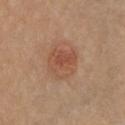follow-up — catalogued during a skin exam; not biopsied | anatomic site — the chest | lighting — white-light illumination | image source — total-body-photography crop, ~15 mm field of view | subject — female, aged 58 to 62 | image-analysis metrics — a footprint of about 11 mm², a shape eccentricity near 0.35, and a symmetry-axis asymmetry near 0.2; a mean CIELAB color near L≈51 a*≈23 b*≈32, about 7 CIELAB-L* units darker than the surrounding skin, and a normalized lesion–skin contrast near 5.5; a classifier nevus-likeness of about 80/100 and lesion-presence confidence of about 100/100 | lesion size — ~4 mm (longest diameter).